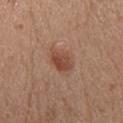No biopsy was performed on this lesion — it was imaged during a full skin examination and was not determined to be concerning. A female patient, in their 70s. From the head or neck. Captured under white-light illumination. Automated image analysis of the tile measured an eccentricity of roughly 0.65 and a shape-asymmetry score of about 0.2 (0 = symmetric). A 15 mm close-up extracted from a 3D total-body photography capture.